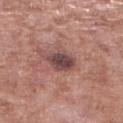Impression:
Part of a total-body skin-imaging series; this lesion was reviewed on a skin check and was not flagged for biopsy.
Context:
The lesion is located on the right lower leg. About 3.5 mm across. This is a white-light tile. The patient is a male in their mid-50s. A 15 mm close-up extracted from a 3D total-body photography capture.